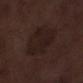Assessment:
The lesion was photographed on a routine skin check and not biopsied; there is no pathology result.
Context:
Measured at roughly 6 mm in maximum diameter. A roughly 15 mm field-of-view crop from a total-body skin photograph. The lesion is on the left lower leg. The lesion-visualizer software estimated a border-irregularity index near 2/10, internal color variation of about 2.5 on a 0–10 scale, and a peripheral color-asymmetry measure near 1. It also reported an automated nevus-likeness rating near 0 out of 100. The subject is a male in their 70s.